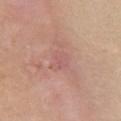| key | value |
|---|---|
| notes | no biopsy performed (imaged during a skin exam) |
| patient | female, in their mid-40s |
| imaging modality | ~15 mm tile from a whole-body skin photo |
| site | the chest |